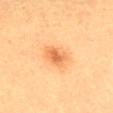Findings:
* workup — total-body-photography surveillance lesion; no biopsy
* site — the mid back
* imaging modality — ~15 mm tile from a whole-body skin photo
* subject — female, approximately 25 years of age